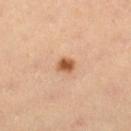follow-up: total-body-photography surveillance lesion; no biopsy | tile lighting: cross-polarized | anatomic site: the leg | lesion size: ≈2 mm | imaging modality: 15 mm crop, total-body photography | patient: male, aged 63 to 67 | automated metrics: an outline eccentricity of about 0.6 (0 = round, 1 = elongated) and two-axis asymmetry of about 0.3; a mean CIELAB color near L≈53 a*≈23 b*≈36, a lesion–skin lightness drop of about 14, and a normalized lesion–skin contrast near 10; a border-irregularity index near 2/10, a within-lesion color-variation index near 2/10, and a peripheral color-asymmetry measure near 0.5; lesion-presence confidence of about 100/100.